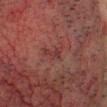Clinical summary:
Captured under cross-polarized illumination. The lesion is located on the head or neck. Longest diameter approximately 2.5 mm. A male subject, aged approximately 60. A 15 mm close-up extracted from a 3D total-body photography capture.